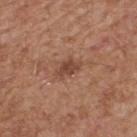A male patient, aged around 65. The recorded lesion diameter is about 3 mm. The tile uses white-light illumination. On the upper back. A roughly 15 mm field-of-view crop from a total-body skin photograph.